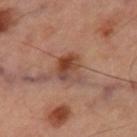Part of a total-body skin-imaging series; this lesion was reviewed on a skin check and was not flagged for biopsy.
The lesion is located on the right thigh.
A 15 mm close-up tile from a total-body photography series done for melanoma screening.
Imaged with cross-polarized lighting.
The recorded lesion diameter is about 3.5 mm.